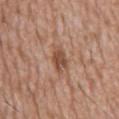A male patient, roughly 60 years of age. From the abdomen. A roughly 15 mm field-of-view crop from a total-body skin photograph. The recorded lesion diameter is about 3.5 mm.